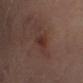biopsy status: imaged on a skin check; not biopsied | lesion diameter: about 2.5 mm | image-analysis metrics: a lesion area of about 3.5 mm², an outline eccentricity of about 0.8 (0 = round, 1 = elongated), and a shape-asymmetry score of about 0.3 (0 = symmetric); a mean CIELAB color near L≈30 a*≈20 b*≈22 and a normalized border contrast of about 6.5; a border-irregularity index near 2.5/10, a within-lesion color-variation index near 1.5/10, and a peripheral color-asymmetry measure near 0.5; a classifier nevus-likeness of about 35/100 | location: the right lower leg | subject: male, aged around 65 | imaging modality: ~15 mm crop, total-body skin-cancer survey | illumination: cross-polarized.